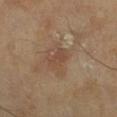Impression:
This lesion was catalogued during total-body skin photography and was not selected for biopsy.
Image and clinical context:
The tile uses cross-polarized illumination. A male subject, in their mid- to late 60s. Located on the right lower leg. A 15 mm crop from a total-body photograph taken for skin-cancer surveillance. Automated image analysis of the tile measured a lesion color around L≈45 a*≈18 b*≈28 in CIELAB, a lesion–skin lightness drop of about 7, and a lesion-to-skin contrast of about 5.5 (normalized; higher = more distinct). And it measured a classifier nevus-likeness of about 0/100 and lesion-presence confidence of about 100/100.